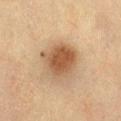| field | value |
|---|---|
| image source | ~15 mm tile from a whole-body skin photo |
| subject | female, in their mid- to late 50s |
| automated lesion analysis | a lesion area of about 15 mm², a shape eccentricity near 0.4, and a symmetry-axis asymmetry near 0.2; a mean CIELAB color near L≈48 a*≈17 b*≈31, roughly 12 lightness units darker than nearby skin, and a normalized lesion–skin contrast near 9; internal color variation of about 4 on a 0–10 scale |
| illumination | cross-polarized illumination |
| anatomic site | the left thigh |
| lesion diameter | ~4.5 mm (longest diameter) |